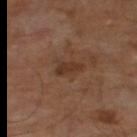Part of a total-body skin-imaging series; this lesion was reviewed on a skin check and was not flagged for biopsy.
The tile uses cross-polarized illumination.
A close-up tile cropped from a whole-body skin photograph, about 15 mm across.
The subject is a male aged 63 to 67.